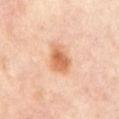{"biopsy_status": "not biopsied; imaged during a skin examination", "patient": {"sex": "female", "age_approx": 50}, "automated_metrics": {"border_irregularity_0_10": 2.0, "color_variation_0_10": 4.0, "peripheral_color_asymmetry": 1.0}, "lesion_size": {"long_diameter_mm_approx": 3.5}, "site": "front of the torso", "lighting": "cross-polarized", "image": {"source": "total-body photography crop", "field_of_view_mm": 15}}